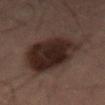Assessment: No biopsy was performed on this lesion — it was imaged during a full skin examination and was not determined to be concerning. Acquisition and patient details: Cropped from a total-body skin-imaging series; the visible field is about 15 mm. The tile uses cross-polarized illumination. Automated image analysis of the tile measured a lesion color around L≈21 a*≈13 b*≈16 in CIELAB, about 11 CIELAB-L* units darker than the surrounding skin, and a normalized lesion–skin contrast near 13. The patient is a male aged 53–57. Longest diameter approximately 9.5 mm. Located on the abdomen.